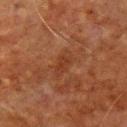{"biopsy_status": "not biopsied; imaged during a skin examination", "patient": {"sex": "male", "age_approx": 80}, "site": "chest", "lighting": "cross-polarized", "automated_metrics": {"border_irregularity_0_10": 3.0, "peripheral_color_asymmetry": 0.5}, "image": {"source": "total-body photography crop", "field_of_view_mm": 15}}